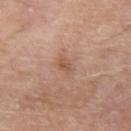Recorded during total-body skin imaging; not selected for excision or biopsy.
A male patient aged 68 to 72.
Located on the right upper arm.
Cropped from a total-body skin-imaging series; the visible field is about 15 mm.